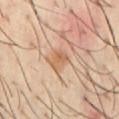Q: Is there a histopathology result?
A: imaged on a skin check; not biopsied
Q: How was the tile lit?
A: cross-polarized
Q: What are the patient's age and sex?
A: male, aged 38 to 42
Q: What did automated image analysis measure?
A: a footprint of about 4.5 mm², an eccentricity of roughly 0.7, and two-axis asymmetry of about 0.25; an average lesion color of about L≈60 a*≈20 b*≈34 (CIELAB) and a normalized border contrast of about 6.5; a detector confidence of about 100 out of 100 that the crop contains a lesion
Q: What kind of image is this?
A: ~15 mm crop, total-body skin-cancer survey
Q: Where on the body is the lesion?
A: the chest